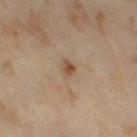Assessment: Captured during whole-body skin photography for melanoma surveillance; the lesion was not biopsied. Background: A region of skin cropped from a whole-body photographic capture, roughly 15 mm wide. An algorithmic analysis of the crop reported an outline eccentricity of about 0.7 (0 = round, 1 = elongated) and a shape-asymmetry score of about 0.4 (0 = symmetric). The software also gave roughly 10 lightness units darker than nearby skin. About 2 mm across. A female patient in their 50s. The lesion is on the right thigh.